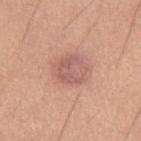biopsy_status: not biopsied; imaged during a skin examination
patient:
  sex: female
  age_approx: 45
automated_metrics:
  area_mm2_approx: 11.0
  eccentricity: 0.4
  shape_asymmetry: 0.15
  cielab_L: 59
  cielab_a: 24
  cielab_b: 25
  vs_skin_darker_L: 9.0
  vs_skin_contrast_norm: 6.0
  color_variation_0_10: 4.0
  peripheral_color_asymmetry: 1.5
site: right thigh
lighting: white-light
image:
  source: total-body photography crop
  field_of_view_mm: 15
lesion_size:
  long_diameter_mm_approx: 4.0